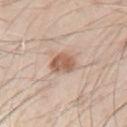patient:
  sex: male
  age_approx: 70
lesion_size:
  long_diameter_mm_approx: 3.5
automated_metrics:
  area_mm2_approx: 6.5
  eccentricity: 0.65
  shape_asymmetry: 0.25
  cielab_L: 59
  cielab_a: 19
  cielab_b: 29
  vs_skin_contrast_norm: 8.5
site: left upper arm
lighting: white-light
image:
  source: total-body photography crop
  field_of_view_mm: 15